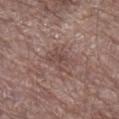Part of a total-body skin-imaging series; this lesion was reviewed on a skin check and was not flagged for biopsy. A male subject, aged around 70. A region of skin cropped from a whole-body photographic capture, roughly 15 mm wide. Imaged with white-light lighting. The recorded lesion diameter is about 3 mm. On the right lower leg.